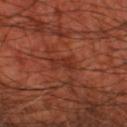<tbp_lesion>
<lighting>cross-polarized</lighting>
<site>leg</site>
<image>
  <source>total-body photography crop</source>
  <field_of_view_mm>15</field_of_view_mm>
</image>
<patient>
  <age_approx>65</age_approx>
</patient>
<automated_metrics>
  <border_irregularity_0_10>4.5</border_irregularity_0_10>
  <color_variation_0_10>2.0</color_variation_0_10>
  <peripheral_color_asymmetry>0.5</peripheral_color_asymmetry>
</automated_metrics>
</tbp_lesion>